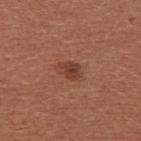biopsy status: imaged on a skin check; not biopsied
automated metrics: a lesion color around L≈40 a*≈24 b*≈28 in CIELAB, a lesion–skin lightness drop of about 9, and a lesion-to-skin contrast of about 7.5 (normalized; higher = more distinct); a border-irregularity index near 2.5/10, a within-lesion color-variation index near 2.5/10, and peripheral color asymmetry of about 1; an automated nevus-likeness rating near 80 out of 100
acquisition: 15 mm crop, total-body photography
anatomic site: the chest
illumination: white-light illumination
diameter: about 2.5 mm
patient: female, approximately 35 years of age